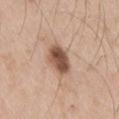| key | value |
|---|---|
| diameter | about 4 mm |
| tile lighting | white-light |
| image-analysis metrics | an area of roughly 9 mm², an outline eccentricity of about 0.7 (0 = round, 1 = elongated), and two-axis asymmetry of about 0.2; a border-irregularity index near 1.5/10, internal color variation of about 5.5 on a 0–10 scale, and radial color variation of about 2; a lesion-detection confidence of about 100/100 |
| body site | the right thigh |
| imaging modality | ~15 mm tile from a whole-body skin photo |
| patient | male, aged 58–62 |